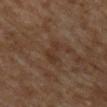Automated tile analysis of the lesion measured a border-irregularity index near 6/10, a within-lesion color-variation index near 1/10, and radial color variation of about 0. The software also gave a nevus-likeness score of about 0/100 and a lesion-detection confidence of about 95/100. Approximately 3.5 mm at its widest. The tile uses cross-polarized illumination. The patient is a female aged approximately 60. On the upper back. This image is a 15 mm lesion crop taken from a total-body photograph.